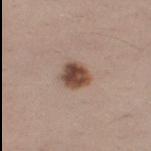Assessment:
Recorded during total-body skin imaging; not selected for excision or biopsy.
Background:
An algorithmic analysis of the crop reported a footprint of about 7 mm² and a symmetry-axis asymmetry near 0.2. And it measured a normalized border contrast of about 11.5. And it measured border irregularity of about 1.5 on a 0–10 scale and radial color variation of about 1.5. It also reported an automated nevus-likeness rating near 100 out of 100 and a detector confidence of about 100 out of 100 that the crop contains a lesion. On the right thigh. Measured at roughly 3 mm in maximum diameter. A 15 mm close-up tile from a total-body photography series done for melanoma screening. A female patient, approximately 45 years of age.